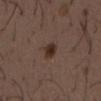body site — the back | image source — ~15 mm tile from a whole-body skin photo | subject — male, aged 48 to 52 | TBP lesion metrics — an area of roughly 4 mm², a shape eccentricity near 0.65, and two-axis asymmetry of about 0.3; a border-irregularity index near 2.5/10, a color-variation rating of about 3/10, and a peripheral color-asymmetry measure near 1 | lesion diameter — ~2.5 mm (longest diameter).